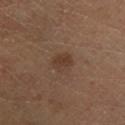biopsy_status: not biopsied; imaged during a skin examination
image:
  source: total-body photography crop
  field_of_view_mm: 15
site: left lower leg
patient:
  sex: female
  age_approx: 55
automated_metrics:
  area_mm2_approx: 4.5
  eccentricity: 0.65
  shape_asymmetry: 0.2
  cielab_L: 38
  cielab_a: 17
  cielab_b: 27
  color_variation_0_10: 2.0
  peripheral_color_asymmetry: 0.5
  nevus_likeness_0_100: 35
  lesion_detection_confidence_0_100: 100
lesion_size:
  long_diameter_mm_approx: 2.5
lighting: cross-polarized